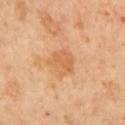The lesion was photographed on a routine skin check and not biopsied; there is no pathology result. The lesion's longest dimension is about 3.5 mm. Imaged with cross-polarized lighting. The patient is a male in their mid-60s. A close-up tile cropped from a whole-body skin photograph, about 15 mm across.